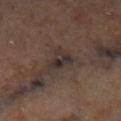| key | value |
|---|---|
| biopsy status | catalogued during a skin exam; not biopsied |
| location | the left lower leg |
| lesion size | about 3.5 mm |
| TBP lesion metrics | an average lesion color of about L≈30 a*≈12 b*≈16 (CIELAB); a border-irregularity index near 4.5/10 and radial color variation of about 2.5 |
| image source | 15 mm crop, total-body photography |
| illumination | cross-polarized |